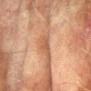Recorded during total-body skin imaging; not selected for excision or biopsy.
A male subject, aged approximately 75.
The recorded lesion diameter is about 2.5 mm.
A roughly 15 mm field-of-view crop from a total-body skin photograph.
Located on the arm.